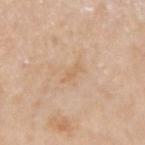Impression: This lesion was catalogued during total-body skin photography and was not selected for biopsy. Background: The lesion is on the right upper arm. A male subject, aged approximately 75. A close-up tile cropped from a whole-body skin photograph, about 15 mm across. Imaged with white-light lighting. An algorithmic analysis of the crop reported an area of roughly 2 mm², an eccentricity of roughly 0.95, and a symmetry-axis asymmetry near 0.3. The software also gave an average lesion color of about L≈64 a*≈17 b*≈36 (CIELAB), a lesion–skin lightness drop of about 6, and a lesion-to-skin contrast of about 5 (normalized; higher = more distinct). It also reported border irregularity of about 4 on a 0–10 scale, a color-variation rating of about 0/10, and a peripheral color-asymmetry measure near 0. About 2.5 mm across.